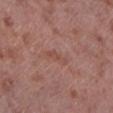Part of a total-body skin-imaging series; this lesion was reviewed on a skin check and was not flagged for biopsy. The lesion is on the left lower leg. A 15 mm crop from a total-body photograph taken for skin-cancer surveillance. Measured at roughly 3 mm in maximum diameter. The patient is a male in their mid- to late 50s.